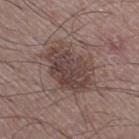workup: imaged on a skin check; not biopsied | subject: male, aged around 50 | image source: 15 mm crop, total-body photography | site: the right thigh.